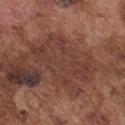Impression:
The lesion was tiled from a total-body skin photograph and was not biopsied.
Acquisition and patient details:
The lesion's longest dimension is about 8 mm. A male patient about 75 years old. The total-body-photography lesion software estimated an average lesion color of about L≈39 a*≈21 b*≈24 (CIELAB), roughly 7 lightness units darker than nearby skin, and a lesion-to-skin contrast of about 6.5 (normalized; higher = more distinct). The analysis additionally found a border-irregularity index near 6.5/10 and internal color variation of about 3 on a 0–10 scale. Captured under white-light illumination. Located on the chest. A 15 mm close-up extracted from a 3D total-body photography capture.